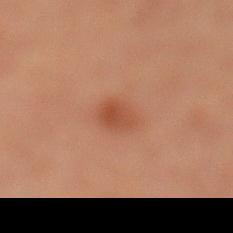No biopsy was performed on this lesion — it was imaged during a full skin examination and was not determined to be concerning. A male patient, approximately 60 years of age. Located on the left lower leg. The lesion's longest dimension is about 2.5 mm. Captured under cross-polarized illumination. Cropped from a whole-body photographic skin survey; the tile spans about 15 mm. Automated image analysis of the tile measured a lesion area of about 3.5 mm² and an outline eccentricity of about 0.65 (0 = round, 1 = elongated). It also reported roughly 7 lightness units darker than nearby skin and a lesion-to-skin contrast of about 6.5 (normalized; higher = more distinct). And it measured a border-irregularity index near 2/10, a color-variation rating of about 2/10, and a peripheral color-asymmetry measure near 0.5.